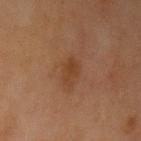Impression:
This lesion was catalogued during total-body skin photography and was not selected for biopsy.
Background:
A female patient approximately 65 years of age. Cropped from a whole-body photographic skin survey; the tile spans about 15 mm. Captured under cross-polarized illumination. Longest diameter approximately 2.5 mm. Located on the left upper arm.